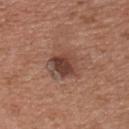Impression:
Part of a total-body skin-imaging series; this lesion was reviewed on a skin check and was not flagged for biopsy.
Clinical summary:
From the chest. An algorithmic analysis of the crop reported a border-irregularity index near 2.5/10, internal color variation of about 5.5 on a 0–10 scale, and peripheral color asymmetry of about 1.5. The software also gave an automated nevus-likeness rating near 85 out of 100 and a detector confidence of about 100 out of 100 that the crop contains a lesion. The patient is a male aged 53 to 57. A lesion tile, about 15 mm wide, cut from a 3D total-body photograph.